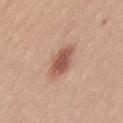biopsy status: imaged on a skin check; not biopsied
patient: female, in their 20s
illumination: white-light
image source: total-body-photography crop, ~15 mm field of view
body site: the left thigh